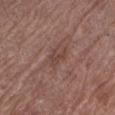{"biopsy_status": "not biopsied; imaged during a skin examination", "site": "left lower leg", "lighting": "white-light", "automated_metrics": {"area_mm2_approx": 3.0, "eccentricity": 0.85, "shape_asymmetry": 0.4, "border_irregularity_0_10": 4.0, "color_variation_0_10": 1.0, "peripheral_color_asymmetry": 0.5}, "patient": {"sex": "female", "age_approx": 80}, "image": {"source": "total-body photography crop", "field_of_view_mm": 15}, "lesion_size": {"long_diameter_mm_approx": 2.5}}